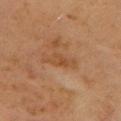Captured during whole-body skin photography for melanoma surveillance; the lesion was not biopsied. A 15 mm close-up extracted from a 3D total-body photography capture. The subject is a female roughly 55 years of age. The total-body-photography lesion software estimated a lesion color around L≈42 a*≈19 b*≈32 in CIELAB, a lesion–skin lightness drop of about 6, and a normalized lesion–skin contrast near 5.5. And it measured an automated nevus-likeness rating near 0 out of 100. From the left upper arm. Captured under cross-polarized illumination.